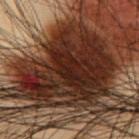The lesion was tiled from a total-body skin photograph and was not biopsied.
The lesion is on the abdomen.
A close-up tile cropped from a whole-body skin photograph, about 15 mm across.
A male subject aged around 55.